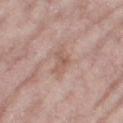Impression:
The lesion was photographed on a routine skin check and not biopsied; there is no pathology result.
Background:
On the right thigh. About 4 mm across. A 15 mm close-up tile from a total-body photography series done for melanoma screening. A female patient, aged around 70. Captured under white-light illumination.